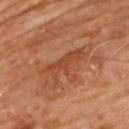Context:
The lesion is located on the upper back. The subject is a male aged around 60. A roughly 15 mm field-of-view crop from a total-body skin photograph.